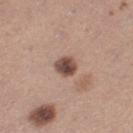biopsy status=catalogued during a skin exam; not biopsied | site=the left thigh | patient=female, aged 28 to 32 | image=~15 mm crop, total-body skin-cancer survey.